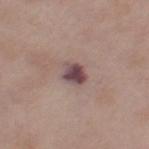Assessment: The lesion was tiled from a total-body skin photograph and was not biopsied. Background: A lesion tile, about 15 mm wide, cut from a 3D total-body photograph. The lesion is on the leg. An algorithmic analysis of the crop reported an outline eccentricity of about 0.5 (0 = round, 1 = elongated) and a shape-asymmetry score of about 0.3 (0 = symmetric). And it measured a classifier nevus-likeness of about 20/100 and a detector confidence of about 100 out of 100 that the crop contains a lesion. The patient is a female about 65 years old. Approximately 2.5 mm at its widest.